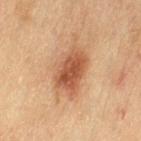  biopsy_status: not biopsied; imaged during a skin examination
  patient:
    sex: female
    age_approx: 55
  site: left thigh
  lighting: cross-polarized
  image:
    source: total-body photography crop
    field_of_view_mm: 15
  lesion_size:
    long_diameter_mm_approx: 6.5